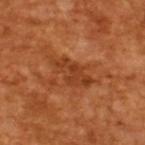<record>
  <biopsy_status>not biopsied; imaged during a skin examination</biopsy_status>
  <lighting>cross-polarized</lighting>
  <image>
    <source>total-body photography crop</source>
    <field_of_view_mm>15</field_of_view_mm>
  </image>
  <patient>
    <sex>male</sex>
    <age_approx>65</age_approx>
  </patient>
  <automated_metrics>
    <area_mm2_approx>9.5</area_mm2_approx>
    <shape_asymmetry>0.3</shape_asymmetry>
    <border_irregularity_0_10>4.5</border_irregularity_0_10>
    <color_variation_0_10>3.0</color_variation_0_10>
    <peripheral_color_asymmetry>1.0</peripheral_color_asymmetry>
    <lesion_detection_confidence_0_100>100</lesion_detection_confidence_0_100>
  </automated_metrics>
</record>